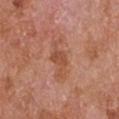Q: Was a biopsy performed?
A: no biopsy performed (imaged during a skin exam)
Q: Illumination type?
A: white-light
Q: What is the anatomic site?
A: the left upper arm
Q: What is the lesion's diameter?
A: ~5 mm (longest diameter)
Q: Who is the patient?
A: male, aged around 65
Q: What did automated image analysis measure?
A: a footprint of about 7 mm², an eccentricity of roughly 0.9, and two-axis asymmetry of about 0.35; a mean CIELAB color near L≈51 a*≈25 b*≈32 and a lesion-to-skin contrast of about 6 (normalized; higher = more distinct); a border-irregularity index near 5.5/10 and a color-variation rating of about 1.5/10; lesion-presence confidence of about 100/100
Q: What is the imaging modality?
A: ~15 mm tile from a whole-body skin photo